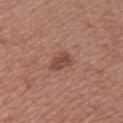Impression: Part of a total-body skin-imaging series; this lesion was reviewed on a skin check and was not flagged for biopsy. Acquisition and patient details: The recorded lesion diameter is about 3 mm. The lesion is on the chest. Captured under white-light illumination. A 15 mm close-up tile from a total-body photography series done for melanoma screening. A female subject aged around 50. An algorithmic analysis of the crop reported a lesion area of about 4.5 mm², a shape eccentricity near 0.75, and two-axis asymmetry of about 0.25. It also reported an average lesion color of about L≈46 a*≈23 b*≈27 (CIELAB) and a normalized lesion–skin contrast near 7. The analysis additionally found a nevus-likeness score of about 65/100.